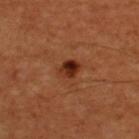Clinical impression:
Recorded during total-body skin imaging; not selected for excision or biopsy.
Acquisition and patient details:
The lesion is on the upper back. A male patient, about 55 years old. A lesion tile, about 15 mm wide, cut from a 3D total-body photograph.